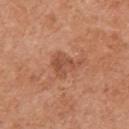Recorded during total-body skin imaging; not selected for excision or biopsy. Cropped from a total-body skin-imaging series; the visible field is about 15 mm. The lesion's longest dimension is about 3.5 mm. The subject is a female in their mid- to late 60s. Automated image analysis of the tile measured an area of roughly 6.5 mm², an outline eccentricity of about 0.65 (0 = round, 1 = elongated), and a symmetry-axis asymmetry near 0.5. And it measured a lesion color around L≈51 a*≈25 b*≈33 in CIELAB and roughly 8 lightness units darker than nearby skin. The software also gave border irregularity of about 5 on a 0–10 scale, a within-lesion color-variation index near 3.5/10, and a peripheral color-asymmetry measure near 1.5. Imaged with white-light lighting. The lesion is on the left arm.